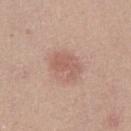Impression: The lesion was tiled from a total-body skin photograph and was not biopsied. Acquisition and patient details: Measured at roughly 3.5 mm in maximum diameter. A female subject approximately 20 years of age. The tile uses white-light illumination. From the left thigh. Cropped from a whole-body photographic skin survey; the tile spans about 15 mm.